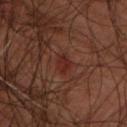{
  "biopsy_status": "not biopsied; imaged during a skin examination",
  "site": "upper back",
  "patient": {
    "sex": "male",
    "age_approx": 45
  },
  "image": {
    "source": "total-body photography crop",
    "field_of_view_mm": 15
  }
}